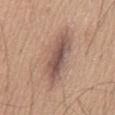Impression: The lesion was photographed on a routine skin check and not biopsied; there is no pathology result. Context: The lesion-visualizer software estimated an average lesion color of about L≈54 a*≈18 b*≈24 (CIELAB) and about 11 CIELAB-L* units darker than the surrounding skin. The software also gave border irregularity of about 4.5 on a 0–10 scale, a within-lesion color-variation index near 6/10, and a peripheral color-asymmetry measure near 2. A 15 mm close-up tile from a total-body photography series done for melanoma screening. The lesion's longest dimension is about 8.5 mm. This is a white-light tile. The patient is a male aged 63 to 67. The lesion is on the abdomen.